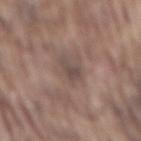Q: Was a biopsy performed?
A: no biopsy performed (imaged during a skin exam)
Q: What is the anatomic site?
A: the mid back
Q: How large is the lesion?
A: ~3.5 mm (longest diameter)
Q: What are the patient's age and sex?
A: male, aged 73 to 77
Q: Illumination type?
A: white-light illumination
Q: What kind of image is this?
A: ~15 mm crop, total-body skin-cancer survey